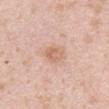Q: Lesion size?
A: ≈3 mm
Q: Lesion location?
A: the right upper arm
Q: Who is the patient?
A: male, about 40 years old
Q: Automated lesion metrics?
A: a lesion color around L≈65 a*≈21 b*≈31 in CIELAB, about 8 CIELAB-L* units darker than the surrounding skin, and a normalized lesion–skin contrast near 6; an automated nevus-likeness rating near 40 out of 100 and lesion-presence confidence of about 100/100
Q: How was this image acquired?
A: 15 mm crop, total-body photography